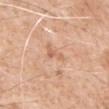notes: no biopsy performed (imaged during a skin exam)
body site: the left upper arm
diameter: ~2.5 mm (longest diameter)
image source: total-body-photography crop, ~15 mm field of view
lighting: white-light
patient: male, roughly 60 years of age